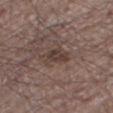biopsy status = catalogued during a skin exam; not biopsied | illumination = white-light illumination | patient = male, aged approximately 65 | location = the right thigh | image-analysis metrics = an area of roughly 5.5 mm² and an eccentricity of roughly 0.8 | lesion diameter = about 3.5 mm | acquisition = 15 mm crop, total-body photography.